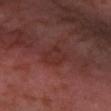Case summary:
– biopsy status · catalogued during a skin exam; not biopsied
– lighting · cross-polarized
– automated lesion analysis · a footprint of about 7.5 mm² and an eccentricity of roughly 0.5; a border-irregularity rating of about 2/10 and radial color variation of about 0.5; a nevus-likeness score of about 0/100 and a lesion-detection confidence of about 85/100
– imaging modality · 15 mm crop, total-body photography
– patient · male, aged approximately 30
– site · the left forearm
– lesion diameter · about 3 mm